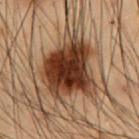workup = catalogued during a skin exam; not biopsied | location = the abdomen | size = ≈7 mm | illumination = cross-polarized illumination | patient = male, in their mid-50s | acquisition = total-body-photography crop, ~15 mm field of view.